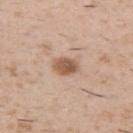Assessment: Recorded during total-body skin imaging; not selected for excision or biopsy. Background: The subject is a male aged 48 to 52. The lesion is located on the left upper arm. Imaged with white-light lighting. The lesion-visualizer software estimated a border-irregularity index near 2/10, a within-lesion color-variation index near 3.5/10, and a peripheral color-asymmetry measure near 1. About 3 mm across. A 15 mm close-up extracted from a 3D total-body photography capture.